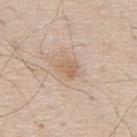Impression: Captured during whole-body skin photography for melanoma surveillance; the lesion was not biopsied. Context: A region of skin cropped from a whole-body photographic capture, roughly 15 mm wide. On the back. A male patient aged 78 to 82. The tile uses white-light illumination. Approximately 2.5 mm at its widest.